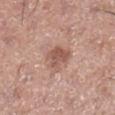image-analysis metrics: a lesion color around L≈55 a*≈21 b*≈27 in CIELAB and a normalized lesion–skin contrast near 7; border irregularity of about 3.5 on a 0–10 scale and radial color variation of about 1; a classifier nevus-likeness of about 45/100 and a detector confidence of about 100 out of 100 that the crop contains a lesion
location: the right lower leg
subject: male, in their mid-50s
image: total-body-photography crop, ~15 mm field of view
diameter: ≈3.5 mm